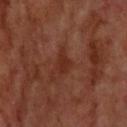No biopsy was performed on this lesion — it was imaged during a full skin examination and was not determined to be concerning.
From the upper back.
Automated image analysis of the tile measured an average lesion color of about L≈29 a*≈24 b*≈28 (CIELAB) and about 7 CIELAB-L* units darker than the surrounding skin.
A 15 mm close-up tile from a total-body photography series done for melanoma screening.
The patient is a male aged around 70.
This is a cross-polarized tile.
Measured at roughly 3 mm in maximum diameter.